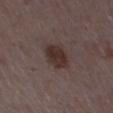* workup · catalogued during a skin exam; not biopsied
* TBP lesion metrics · a shape eccentricity near 0.8 and two-axis asymmetry of about 0.15; an average lesion color of about L≈30 a*≈15 b*≈17 (CIELAB) and a normalized border contrast of about 9.5; a classifier nevus-likeness of about 80/100 and a detector confidence of about 100 out of 100 that the crop contains a lesion
* location · the leg
* imaging modality · ~15 mm tile from a whole-body skin photo
* patient · female, aged approximately 35
* lighting · white-light
* size · about 4 mm